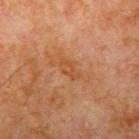Imaged during a routine full-body skin examination; the lesion was not biopsied and no histopathology is available. A 15 mm close-up extracted from a 3D total-body photography capture. On the left upper arm. A male patient, aged around 80. Longest diameter approximately 3 mm.